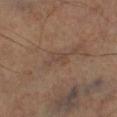Q: Is there a histopathology result?
A: imaged on a skin check; not biopsied
Q: Who is the patient?
A: male, about 60 years old
Q: Where on the body is the lesion?
A: the right lower leg
Q: How large is the lesion?
A: ≈3 mm
Q: How was the tile lit?
A: cross-polarized
Q: What is the imaging modality?
A: 15 mm crop, total-body photography
Q: Automated lesion metrics?
A: an average lesion color of about L≈41 a*≈16 b*≈24 (CIELAB), a lesion–skin lightness drop of about 5, and a normalized border contrast of about 5; a border-irregularity rating of about 5/10